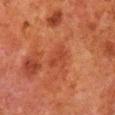Q: Is there a histopathology result?
A: no biopsy performed (imaged during a skin exam)
Q: Who is the patient?
A: male, roughly 80 years of age
Q: Where on the body is the lesion?
A: the right lower leg
Q: What kind of image is this?
A: total-body-photography crop, ~15 mm field of view
Q: What did automated image analysis measure?
A: a mean CIELAB color near L≈35 a*≈27 b*≈30, a lesion–skin lightness drop of about 5, and a normalized lesion–skin contrast near 5